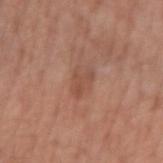Approximately 3 mm at its widest. Captured under white-light illumination. Located on the right forearm. Cropped from a whole-body photographic skin survey; the tile spans about 15 mm. An algorithmic analysis of the crop reported a lesion-detection confidence of about 100/100. The subject is a female aged 48–52.